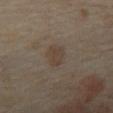Q: Was this lesion biopsied?
A: catalogued during a skin exam; not biopsied
Q: What is the lesion's diameter?
A: about 3 mm
Q: What kind of image is this?
A: total-body-photography crop, ~15 mm field of view
Q: Where on the body is the lesion?
A: the right forearm
Q: Who is the patient?
A: female, roughly 35 years of age